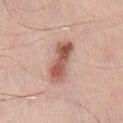<tbp_lesion>
  <biopsy_status>not biopsied; imaged during a skin examination</biopsy_status>
  <site>chest</site>
  <patient>
    <sex>male</sex>
    <age_approx>45</age_approx>
  </patient>
  <lesion_size>
    <long_diameter_mm_approx>5.0</long_diameter_mm_approx>
  </lesion_size>
  <automated_metrics>
    <area_mm2_approx>10.0</area_mm2_approx>
    <shape_asymmetry>0.2</shape_asymmetry>
    <vs_skin_darker_L>15.0</vs_skin_darker_L>
    <vs_skin_contrast_norm>9.5</vs_skin_contrast_norm>
    <nevus_likeness_0_100>90</nevus_likeness_0_100>
  </automated_metrics>
  <lighting>white-light</lighting>
  <image>
    <source>total-body photography crop</source>
    <field_of_view_mm>15</field_of_view_mm>
  </image>
</tbp_lesion>